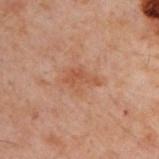Clinical impression:
The lesion was tiled from a total-body skin photograph and was not biopsied.
Context:
A male patient, about 50 years old. A 15 mm close-up extracted from a 3D total-body photography capture. The lesion is on the upper back.